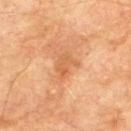notes = catalogued during a skin exam; not biopsied | acquisition = 15 mm crop, total-body photography | patient = male, aged approximately 75 | site = the upper back | illumination = cross-polarized illumination.